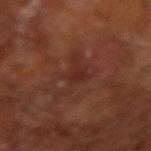Assessment:
No biopsy was performed on this lesion — it was imaged during a full skin examination and was not determined to be concerning.
Clinical summary:
Automated image analysis of the tile measured an eccentricity of roughly 0.8 and two-axis asymmetry of about 0.6. And it measured a lesion–skin lightness drop of about 5 and a normalized lesion–skin contrast near 5.5. And it measured an automated nevus-likeness rating near 0 out of 100. This image is a 15 mm lesion crop taken from a total-body photograph. The recorded lesion diameter is about 5.5 mm. Imaged with cross-polarized lighting. The subject is a male aged 63 to 67.